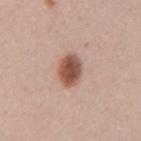<record>
<biopsy_status>not biopsied; imaged during a skin examination</biopsy_status>
<patient>
  <sex>female</sex>
  <age_approx>25</age_approx>
</patient>
<lesion_size>
  <long_diameter_mm_approx>4.0</long_diameter_mm_approx>
</lesion_size>
<lighting>white-light</lighting>
<site>right upper arm</site>
<image>
  <source>total-body photography crop</source>
  <field_of_view_mm>15</field_of_view_mm>
</image>
<automated_metrics>
  <area_mm2_approx>8.0</area_mm2_approx>
  <eccentricity>0.7</eccentricity>
  <shape_asymmetry>0.25</shape_asymmetry>
  <border_irregularity_0_10>2.5</border_irregularity_0_10>
  <color_variation_0_10>4.5</color_variation_0_10>
  <peripheral_color_asymmetry>1.5</peripheral_color_asymmetry>
  <nevus_likeness_0_100>100</nevus_likeness_0_100>
  <lesion_detection_confidence_0_100>100</lesion_detection_confidence_0_100>
</automated_metrics>
</record>